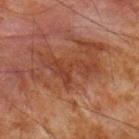{
  "biopsy_status": "not biopsied; imaged during a skin examination",
  "patient": {
    "sex": "male",
    "age_approx": 65
  },
  "site": "upper back",
  "image": {
    "source": "total-body photography crop",
    "field_of_view_mm": 15
  }
}